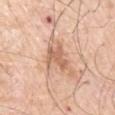Notes:
– tile lighting — white-light
– anatomic site — the left upper arm
– lesion diameter — ~3.5 mm (longest diameter)
– patient — male, roughly 70 years of age
– imaging modality — total-body-photography crop, ~15 mm field of view
– automated lesion analysis — an eccentricity of roughly 0.75 and two-axis asymmetry of about 0.4; a mean CIELAB color near L≈63 a*≈22 b*≈32, about 11 CIELAB-L* units darker than the surrounding skin, and a normalized lesion–skin contrast near 7; a border-irregularity rating of about 5/10, a color-variation rating of about 2.5/10, and radial color variation of about 0.5; a nevus-likeness score of about 0/100 and lesion-presence confidence of about 100/100